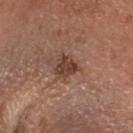The lesion was tiled from a total-body skin photograph and was not biopsied. On the head or neck. This image is a 15 mm lesion crop taken from a total-body photograph. A male patient, roughly 40 years of age.